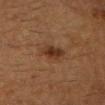This lesion was catalogued during total-body skin photography and was not selected for biopsy. A 15 mm close-up extracted from a 3D total-body photography capture. The tile uses cross-polarized illumination. Longest diameter approximately 3 mm. A female patient, aged 48 to 52. The lesion is located on the head or neck. Automated tile analysis of the lesion measured an area of roughly 5.5 mm², an outline eccentricity of about 0.75 (0 = round, 1 = elongated), and a symmetry-axis asymmetry near 0.2. The software also gave a lesion color around L≈27 a*≈16 b*≈26 in CIELAB, a lesion–skin lightness drop of about 8, and a normalized lesion–skin contrast near 8.5. It also reported border irregularity of about 2 on a 0–10 scale, internal color variation of about 3 on a 0–10 scale, and peripheral color asymmetry of about 1. The analysis additionally found a nevus-likeness score of about 85/100 and a lesion-detection confidence of about 100/100.